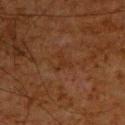The lesion was photographed on a routine skin check and not biopsied; there is no pathology result. Captured under cross-polarized illumination. A lesion tile, about 15 mm wide, cut from a 3D total-body photograph. Measured at roughly 2.5 mm in maximum diameter. A male subject about 65 years old. Located on the upper back.